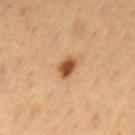Clinical impression: Part of a total-body skin-imaging series; this lesion was reviewed on a skin check and was not flagged for biopsy. Context: The patient is a male aged 48 to 52. The lesion is on the back. The recorded lesion diameter is about 3 mm. Cropped from a total-body skin-imaging series; the visible field is about 15 mm.